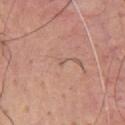site: chest
lighting: white-light
image:
  source: total-body photography crop
  field_of_view_mm: 15
lesion_size:
  long_diameter_mm_approx: 1.0
patient:
  sex: male
  age_approx: 55